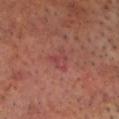Clinical impression:
This lesion was catalogued during total-body skin photography and was not selected for biopsy.
Image and clinical context:
Automated image analysis of the tile measured a footprint of about 4 mm² and an eccentricity of roughly 0.6. The software also gave a border-irregularity rating of about 5/10 and peripheral color asymmetry of about 0.5. The lesion's longest dimension is about 3 mm. A male subject approximately 65 years of age. A 15 mm close-up tile from a total-body photography series done for melanoma screening. The lesion is on the head or neck.